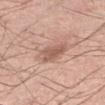Clinical impression:
No biopsy was performed on this lesion — it was imaged during a full skin examination and was not determined to be concerning.
Acquisition and patient details:
Captured under white-light illumination. A male subject aged 38 to 42. On the left lower leg. Measured at roughly 4.5 mm in maximum diameter. A region of skin cropped from a whole-body photographic capture, roughly 15 mm wide.